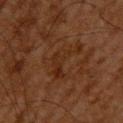Impression:
The lesion was photographed on a routine skin check and not biopsied; there is no pathology result.
Context:
The lesion is located on the upper back. The lesion's longest dimension is about 5.5 mm. Automated tile analysis of the lesion measured roughly 4 lightness units darker than nearby skin and a normalized border contrast of about 5.5. A male subject aged around 65. Cropped from a whole-body photographic skin survey; the tile spans about 15 mm.